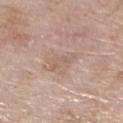<lesion>
<biopsy_status>not biopsied; imaged during a skin examination</biopsy_status>
<patient>
  <sex>female</sex>
  <age_approx>75</age_approx>
</patient>
<lighting>white-light</lighting>
<site>right lower leg</site>
<automated_metrics>
  <area_mm2_approx>4.0</area_mm2_approx>
  <eccentricity>0.9</eccentricity>
  <border_irregularity_0_10>5.5</border_irregularity_0_10>
  <color_variation_0_10>1.0</color_variation_0_10>
  <peripheral_color_asymmetry>0.5</peripheral_color_asymmetry>
  <nevus_likeness_0_100>0</nevus_likeness_0_100>
  <lesion_detection_confidence_0_100>100</lesion_detection_confidence_0_100>
</automated_metrics>
<image>
  <source>total-body photography crop</source>
  <field_of_view_mm>15</field_of_view_mm>
</image>
</lesion>